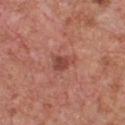Impression: Imaged during a routine full-body skin examination; the lesion was not biopsied and no histopathology is available. Image and clinical context: On the chest. A male subject aged around 65. Cropped from a whole-body photographic skin survey; the tile spans about 15 mm.